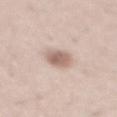The lesion was tiled from a total-body skin photograph and was not biopsied.
Imaged with white-light lighting.
Located on the mid back.
Approximately 3.5 mm at its widest.
A male patient approximately 35 years of age.
Automated image analysis of the tile measured an area of roughly 7 mm², a shape eccentricity near 0.7, and two-axis asymmetry of about 0.15.
A 15 mm close-up extracted from a 3D total-body photography capture.